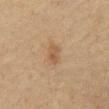This lesion was catalogued during total-body skin photography and was not selected for biopsy. Cropped from a whole-body photographic skin survey; the tile spans about 15 mm. From the abdomen. A male patient aged 58–62. The tile uses cross-polarized illumination. Approximately 3 mm at its widest.